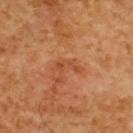<record>
  <biopsy_status>not biopsied; imaged during a skin examination</biopsy_status>
  <site>upper back</site>
  <image>
    <source>total-body photography crop</source>
    <field_of_view_mm>15</field_of_view_mm>
  </image>
  <patient>
    <sex>male</sex>
    <age_approx>60</age_approx>
  </patient>
</record>